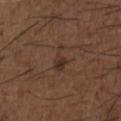Q: Was this lesion biopsied?
A: no biopsy performed (imaged during a skin exam)
Q: How was the tile lit?
A: white-light illumination
Q: What are the patient's age and sex?
A: male, aged around 50
Q: What kind of image is this?
A: 15 mm crop, total-body photography
Q: Lesion size?
A: ≈3.5 mm
Q: What did automated image analysis measure?
A: a shape eccentricity near 0.85; a nevus-likeness score of about 0/100
Q: Where on the body is the lesion?
A: the front of the torso